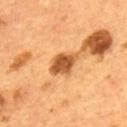notes: total-body-photography surveillance lesion; no biopsy
size: ~4 mm (longest diameter)
image source: 15 mm crop, total-body photography
location: the upper back
lighting: cross-polarized illumination
subject: male, in their mid-50s
automated metrics: a mean CIELAB color near L≈51 a*≈25 b*≈41, a lesion–skin lightness drop of about 16, and a lesion-to-skin contrast of about 10.5 (normalized; higher = more distinct)